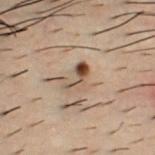{
  "biopsy_status": "not biopsied; imaged during a skin examination",
  "image": {
    "source": "total-body photography crop",
    "field_of_view_mm": 15
  },
  "patient": {
    "sex": "male",
    "age_approx": 30
  },
  "lighting": "cross-polarized",
  "lesion_size": {
    "long_diameter_mm_approx": 3.5
  },
  "site": "chest"
}